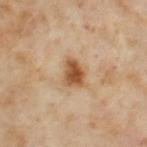Impression:
Captured during whole-body skin photography for melanoma surveillance; the lesion was not biopsied.
Background:
The recorded lesion diameter is about 3 mm. A female subject, in their mid-50s. The tile uses cross-polarized illumination. Cropped from a whole-body photographic skin survey; the tile spans about 15 mm. The total-body-photography lesion software estimated an area of roughly 6.5 mm², a shape eccentricity near 0.7, and a symmetry-axis asymmetry near 0.25. The lesion is located on the leg.